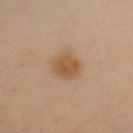The lesion was tiled from a total-body skin photograph and was not biopsied. Approximately 3.5 mm at its widest. The subject is a female aged 58 to 62. The tile uses cross-polarized illumination. Cropped from a total-body skin-imaging series; the visible field is about 15 mm. Located on the chest.